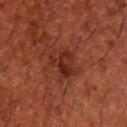Captured during whole-body skin photography for melanoma surveillance; the lesion was not biopsied. The lesion-visualizer software estimated border irregularity of about 4 on a 0–10 scale, a color-variation rating of about 5/10, and peripheral color asymmetry of about 2. The software also gave a nevus-likeness score of about 45/100 and a detector confidence of about 100 out of 100 that the crop contains a lesion. Cropped from a total-body skin-imaging series; the visible field is about 15 mm. Imaged with cross-polarized lighting. The patient is a male about 50 years old. Located on the upper back.